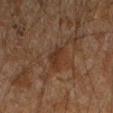Notes:
* TBP lesion metrics — a lesion area of about 3.5 mm², an eccentricity of roughly 0.85, and a shape-asymmetry score of about 0.4 (0 = symmetric); a lesion–skin lightness drop of about 6 and a normalized border contrast of about 6.5; radial color variation of about 0.5
* patient — male, aged 43–47
* lesion size — about 3 mm
* anatomic site — the arm
* image source — ~15 mm tile from a whole-body skin photo